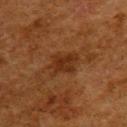biopsy status: catalogued during a skin exam; not biopsied
lesion diameter: about 4 mm
lighting: cross-polarized illumination
anatomic site: the upper back
patient: female, about 50 years old
image source: 15 mm crop, total-body photography